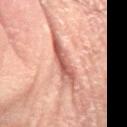Findings:
* biopsy status: total-body-photography surveillance lesion; no biopsy
* illumination: cross-polarized
* image-analysis metrics: an average lesion color of about L≈52 a*≈25 b*≈26 (CIELAB) and a normalized lesion–skin contrast near 8.5
* lesion size: ≈7 mm
* imaging modality: ~15 mm tile from a whole-body skin photo
* patient: male, aged 53 to 57
* site: the right forearm